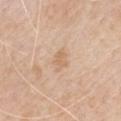Notes:
• workup — total-body-photography surveillance lesion; no biopsy
• subject — male, aged around 65
• lighting — white-light illumination
• TBP lesion metrics — an area of roughly 3.5 mm², a shape eccentricity near 0.75, and a shape-asymmetry score of about 0.25 (0 = symmetric)
• anatomic site — the chest
• image source — ~15 mm tile from a whole-body skin photo